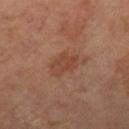The lesion was tiled from a total-body skin photograph and was not biopsied. The recorded lesion diameter is about 3.5 mm. A 15 mm close-up extracted from a 3D total-body photography capture. A female subject, roughly 65 years of age. The tile uses cross-polarized illumination. An algorithmic analysis of the crop reported an area of roughly 6 mm², an eccentricity of roughly 0.75, and a shape-asymmetry score of about 0.25 (0 = symmetric). The analysis additionally found a classifier nevus-likeness of about 0/100 and a detector confidence of about 100 out of 100 that the crop contains a lesion. The lesion is located on the right thigh.